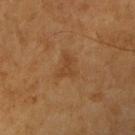Assessment: Part of a total-body skin-imaging series; this lesion was reviewed on a skin check and was not flagged for biopsy. Background: Measured at roughly 3 mm in maximum diameter. A male subject, about 60 years old. An algorithmic analysis of the crop reported a classifier nevus-likeness of about 0/100 and a detector confidence of about 100 out of 100 that the crop contains a lesion. This is a cross-polarized tile. The lesion is on the right upper arm. A 15 mm close-up tile from a total-body photography series done for melanoma screening.